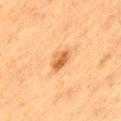Context: The tile uses cross-polarized illumination. Longest diameter approximately 2.5 mm. The total-body-photography lesion software estimated an average lesion color of about L≈51 a*≈22 b*≈37 (CIELAB), about 11 CIELAB-L* units darker than the surrounding skin, and a normalized border contrast of about 8. The analysis additionally found a within-lesion color-variation index near 3/10. The software also gave a nevus-likeness score of about 90/100 and a lesion-detection confidence of about 100/100. A male patient approximately 75 years of age. The lesion is on the left thigh. A 15 mm crop from a total-body photograph taken for skin-cancer surveillance.